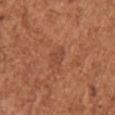The lesion was photographed on a routine skin check and not biopsied; there is no pathology result.
Located on the right upper arm.
Automated tile analysis of the lesion measured a footprint of about 3.5 mm² and an eccentricity of roughly 0.8. It also reported a mean CIELAB color near L≈46 a*≈25 b*≈32 and roughly 6 lightness units darker than nearby skin. The analysis additionally found a border-irregularity index near 2.5/10 and peripheral color asymmetry of about 1. The software also gave lesion-presence confidence of about 100/100.
The lesion's longest dimension is about 2.5 mm.
Cropped from a total-body skin-imaging series; the visible field is about 15 mm.
The patient is a female aged 48 to 52.
Imaged with white-light lighting.